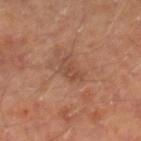Part of a total-body skin-imaging series; this lesion was reviewed on a skin check and was not flagged for biopsy.
A 15 mm crop from a total-body photograph taken for skin-cancer surveillance.
From the right lower leg.
The tile uses cross-polarized illumination.
A male subject, in their mid- to late 60s.
Automated tile analysis of the lesion measured a border-irregularity rating of about 3.5/10, internal color variation of about 3 on a 0–10 scale, and peripheral color asymmetry of about 1. It also reported a nevus-likeness score of about 0/100 and lesion-presence confidence of about 100/100.